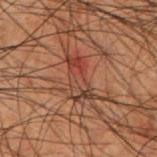  lighting: cross-polarized
  image:
    source: total-body photography crop
    field_of_view_mm: 15
  patient:
    sex: male
    age_approx: 50
  site: right forearm
  lesion_size:
    long_diameter_mm_approx: 4.5